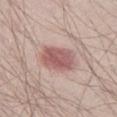No biopsy was performed on this lesion — it was imaged during a full skin examination and was not determined to be concerning.
A male subject, aged approximately 40.
Automated image analysis of the tile measured a lesion color around L≈56 a*≈23 b*≈21 in CIELAB, roughly 12 lightness units darker than nearby skin, and a normalized lesion–skin contrast near 8.
This image is a 15 mm lesion crop taken from a total-body photograph.
On the leg.